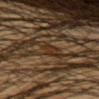Assessment: The lesion was tiled from a total-body skin photograph and was not biopsied. Background: The subject is a male aged 43–47. The lesion is located on the left forearm. A 15 mm close-up tile from a total-body photography series done for melanoma screening.